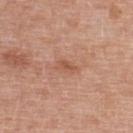notes: imaged on a skin check; not biopsied | lesion size: ~2.5 mm (longest diameter) | patient: female, roughly 40 years of age | imaging modality: ~15 mm tile from a whole-body skin photo | site: the upper back.